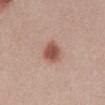Findings:
- tile lighting — white-light
- acquisition — ~15 mm tile from a whole-body skin photo
- body site — the front of the torso
- patient — female, in their mid- to late 20s
- image-analysis metrics — an area of roughly 7 mm², an outline eccentricity of about 0.75 (0 = round, 1 = elongated), and a symmetry-axis asymmetry near 0.15; an average lesion color of about L≈53 a*≈23 b*≈27 (CIELAB); a detector confidence of about 100 out of 100 that the crop contains a lesion
- lesion diameter — ≈3.5 mm